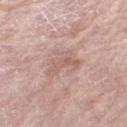<lesion>
<biopsy_status>not biopsied; imaged during a skin examination</biopsy_status>
<patient>
  <sex>female</sex>
  <age_approx>70</age_approx>
</patient>
<lesion_size>
  <long_diameter_mm_approx>4.5</long_diameter_mm_approx>
</lesion_size>
<image>
  <source>total-body photography crop</source>
  <field_of_view_mm>15</field_of_view_mm>
</image>
<site>left thigh</site>
<automated_metrics>
  <cielab_L>60</cielab_L>
  <cielab_a>19</cielab_a>
  <cielab_b>24</cielab_b>
  <vs_skin_darker_L>8.0</vs_skin_darker_L>
  <vs_skin_contrast_norm>5.5</vs_skin_contrast_norm>
  <border_irregularity_0_10>6.5</border_irregularity_0_10>
  <peripheral_color_asymmetry>1.0</peripheral_color_asymmetry>
  <nevus_likeness_0_100>0</nevus_likeness_0_100>
  <lesion_detection_confidence_0_100>100</lesion_detection_confidence_0_100>
</automated_metrics>
</lesion>